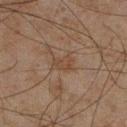Q: Was this lesion biopsied?
A: imaged on a skin check; not biopsied
Q: Where on the body is the lesion?
A: the right lower leg
Q: How was this image acquired?
A: ~15 mm crop, total-body skin-cancer survey
Q: What are the patient's age and sex?
A: male, roughly 45 years of age
Q: What lighting was used for the tile?
A: cross-polarized
Q: Lesion size?
A: about 3 mm